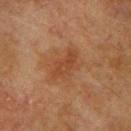Assessment: The lesion was tiled from a total-body skin photograph and was not biopsied. Clinical summary: A male subject, in their mid-70s. From the back. About 4 mm across. Automated image analysis of the tile measured a shape eccentricity near 0.75 and two-axis asymmetry of about 0.3. Imaged with cross-polarized lighting. A 15 mm crop from a total-body photograph taken for skin-cancer surveillance.